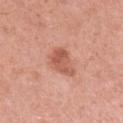  biopsy_status: not biopsied; imaged during a skin examination
  lighting: white-light
  automated_metrics:
    vs_skin_darker_L: 10.0
  site: left upper arm
  patient:
    sex: female
    age_approx: 35
  lesion_size:
    long_diameter_mm_approx: 4.0
  image:
    source: total-body photography crop
    field_of_view_mm: 15